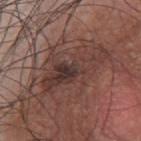Assessment:
Captured during whole-body skin photography for melanoma surveillance; the lesion was not biopsied.
Acquisition and patient details:
This image is a 15 mm lesion crop taken from a total-body photograph. The lesion's longest dimension is about 9.5 mm. This is a white-light tile. The lesion is on the front of the torso. The subject is a male in their mid-40s.